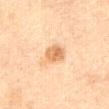Findings:
- patient · female, in their mid- to late 50s
- imaging modality · ~15 mm tile from a whole-body skin photo
- lighting · cross-polarized illumination
- TBP lesion metrics · an outline eccentricity of about 0.7 (0 = round, 1 = elongated) and a symmetry-axis asymmetry near 0.2; a lesion color around L≈61 a*≈19 b*≈37 in CIELAB and roughly 11 lightness units darker than nearby skin; a border-irregularity index near 1.5/10, a color-variation rating of about 4/10, and peripheral color asymmetry of about 1; a classifier nevus-likeness of about 65/100 and a detector confidence of about 100 out of 100 that the crop contains a lesion
- body site · the front of the torso